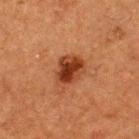Captured during whole-body skin photography for melanoma surveillance; the lesion was not biopsied.
A close-up tile cropped from a whole-body skin photograph, about 15 mm across.
From the upper back.
A male subject about 50 years old.